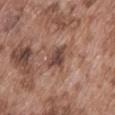{"biopsy_status": "not biopsied; imaged during a skin examination", "site": "abdomen", "patient": {"sex": "male", "age_approx": 75}, "image": {"source": "total-body photography crop", "field_of_view_mm": 15}}